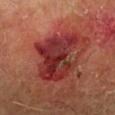Q: Was this lesion biopsied?
A: no biopsy performed (imaged during a skin exam)
Q: How was the tile lit?
A: cross-polarized
Q: What kind of image is this?
A: total-body-photography crop, ~15 mm field of view
Q: What is the anatomic site?
A: the right lower leg
Q: Who is the patient?
A: male, roughly 60 years of age